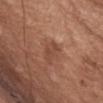A 15 mm close-up tile from a total-body photography series done for melanoma screening. Located on the abdomen. A female patient aged approximately 65.